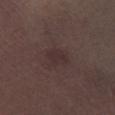Assessment: The lesion was tiled from a total-body skin photograph and was not biopsied. Acquisition and patient details: A male patient, in their mid-50s. Imaged with white-light lighting. Cropped from a total-body skin-imaging series; the visible field is about 15 mm. From the right lower leg. Approximately 2.5 mm at its widest. The lesion-visualizer software estimated a lesion area of about 4 mm², an eccentricity of roughly 0.7, and a shape-asymmetry score of about 0.25 (0 = symmetric). And it measured an average lesion color of about L≈29 a*≈15 b*≈14 (CIELAB) and a normalized lesion–skin contrast near 5.5. The software also gave a color-variation rating of about 1/10 and a peripheral color-asymmetry measure near 0.5.